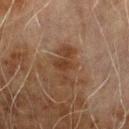Captured during whole-body skin photography for melanoma surveillance; the lesion was not biopsied. Automated tile analysis of the lesion measured a footprint of about 8 mm², a shape eccentricity near 0.9, and a symmetry-axis asymmetry near 0.45. And it measured a mean CIELAB color near L≈32 a*≈17 b*≈26 and roughly 7 lightness units darker than nearby skin. And it measured a border-irregularity rating of about 7/10, a color-variation rating of about 1.5/10, and peripheral color asymmetry of about 0.5. The software also gave a lesion-detection confidence of about 100/100. A male patient roughly 70 years of age. A region of skin cropped from a whole-body photographic capture, roughly 15 mm wide. The lesion is located on the right upper arm. The lesion's longest dimension is about 5 mm. Imaged with cross-polarized lighting.